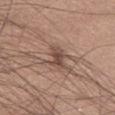This lesion was catalogued during total-body skin photography and was not selected for biopsy.
This is a white-light tile.
A region of skin cropped from a whole-body photographic capture, roughly 15 mm wide.
The subject is a male approximately 60 years of age.
About 3.5 mm across.
On the chest.
An algorithmic analysis of the crop reported a border-irregularity index near 3.5/10, a color-variation rating of about 2.5/10, and radial color variation of about 1. It also reported an automated nevus-likeness rating near 5 out of 100 and lesion-presence confidence of about 90/100.